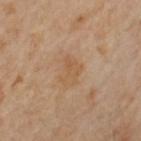Assessment:
This lesion was catalogued during total-body skin photography and was not selected for biopsy.
Background:
On the left upper arm. A female patient, in their mid- to late 50s. The lesion-visualizer software estimated an outline eccentricity of about 0.75 (0 = round, 1 = elongated). The software also gave an average lesion color of about L≈56 a*≈18 b*≈35 (CIELAB), a lesion–skin lightness drop of about 6, and a normalized lesion–skin contrast near 5.5. The analysis additionally found a border-irregularity rating of about 6/10 and radial color variation of about 0.5. A close-up tile cropped from a whole-body skin photograph, about 15 mm across. Captured under cross-polarized illumination. Approximately 3 mm at its widest.